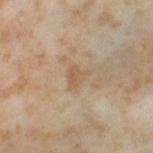{"biopsy_status": "not biopsied; imaged during a skin examination", "patient": {"sex": "female", "age_approx": 55}, "image": {"source": "total-body photography crop", "field_of_view_mm": 15}, "lighting": "cross-polarized", "lesion_size": {"long_diameter_mm_approx": 3.0}, "site": "leg"}